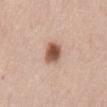Notes:
* notes · total-body-photography surveillance lesion; no biopsy
* tile lighting · white-light illumination
* size · about 3.5 mm
* subject · male, aged around 50
* body site · the abdomen
* imaging modality · ~15 mm crop, total-body skin-cancer survey